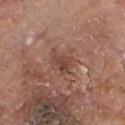A 15 mm crop from a total-body photograph taken for skin-cancer surveillance. From the chest. The subject is a male aged approximately 80.